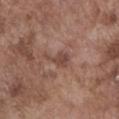Clinical impression: This lesion was catalogued during total-body skin photography and was not selected for biopsy. Image and clinical context: This is a white-light tile. A male patient, aged around 75. The lesion is located on the front of the torso. A 15 mm close-up extracted from a 3D total-body photography capture.